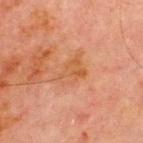| feature | finding |
|---|---|
| body site | the chest |
| subject | male, aged approximately 70 |
| image | 15 mm crop, total-body photography |
| illumination | cross-polarized |
| automated lesion analysis | an area of roughly 7 mm² and a symmetry-axis asymmetry near 0.45; an average lesion color of about L≈47 a*≈22 b*≈33 (CIELAB) and a normalized border contrast of about 5.5 |